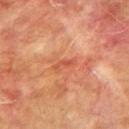follow-up: catalogued during a skin exam; not biopsied
diameter: about 3 mm
location: the right upper arm
subject: male, aged 73 to 77
automated metrics: a border-irregularity rating of about 4.5/10, a within-lesion color-variation index near 0/10, and peripheral color asymmetry of about 0
illumination: cross-polarized
image source: ~15 mm crop, total-body skin-cancer survey